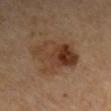Part of a total-body skin-imaging series; this lesion was reviewed on a skin check and was not flagged for biopsy. This is a cross-polarized tile. The lesion-visualizer software estimated a footprint of about 23 mm², a shape eccentricity near 0.6, and a symmetry-axis asymmetry near 0.2. And it measured roughly 9 lightness units darker than nearby skin and a normalized border contrast of about 8.5. It also reported a border-irregularity index near 2.5/10 and peripheral color asymmetry of about 3.5. The lesion is on the right upper arm. The subject is in their mid-60s. A roughly 15 mm field-of-view crop from a total-body skin photograph. About 6 mm across.